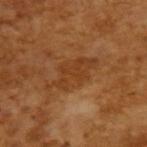Q: Was this lesion biopsied?
A: catalogued during a skin exam; not biopsied
Q: Patient demographics?
A: male, aged around 65
Q: What kind of image is this?
A: 15 mm crop, total-body photography
Q: Lesion size?
A: ≈6 mm